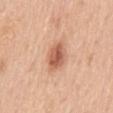Captured during whole-body skin photography for melanoma surveillance; the lesion was not biopsied. The lesion is located on the mid back. The lesion's longest dimension is about 3.5 mm. A lesion tile, about 15 mm wide, cut from a 3D total-body photograph. Imaged with white-light lighting. A male subject, aged around 50. Automated image analysis of the tile measured a shape eccentricity near 0.65 and two-axis asymmetry of about 0.1. The software also gave an average lesion color of about L≈59 a*≈25 b*≈33 (CIELAB) and a lesion-to-skin contrast of about 8 (normalized; higher = more distinct).